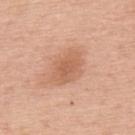Recorded during total-body skin imaging; not selected for excision or biopsy. A lesion tile, about 15 mm wide, cut from a 3D total-body photograph. This is a white-light tile. A male subject, in their mid-70s. Measured at roughly 4.5 mm in maximum diameter. From the upper back.